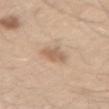The lesion was photographed on a routine skin check and not biopsied; there is no pathology result. From the leg. A close-up tile cropped from a whole-body skin photograph, about 15 mm across. This is a white-light tile. A male subject roughly 60 years of age.